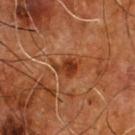Imaged during a routine full-body skin examination; the lesion was not biopsied and no histopathology is available.
From the chest.
The recorded lesion diameter is about 3.5 mm.
A lesion tile, about 15 mm wide, cut from a 3D total-body photograph.
The patient is a male about 55 years old.
This is a cross-polarized tile.